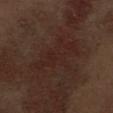Case summary:
* workup — no biopsy performed (imaged during a skin exam)
* patient — male, aged 68 to 72
* image source — ~15 mm tile from a whole-body skin photo
* illumination — white-light
* body site — the abdomen
* automated lesion analysis — border irregularity of about 8 on a 0–10 scale, a color-variation rating of about 2/10, and peripheral color asymmetry of about 0.5